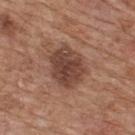follow-up = no biopsy performed (imaged during a skin exam); site = the upper back; acquisition = 15 mm crop, total-body photography; patient = male, aged approximately 65.